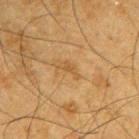Q: Was a biopsy performed?
A: catalogued during a skin exam; not biopsied
Q: Lesion size?
A: ~2.5 mm (longest diameter)
Q: What kind of image is this?
A: 15 mm crop, total-body photography
Q: Who is the patient?
A: male, aged 63–67
Q: Automated lesion metrics?
A: an area of roughly 2.5 mm²; roughly 6 lightness units darker than nearby skin; lesion-presence confidence of about 100/100
Q: Where on the body is the lesion?
A: the right upper arm
Q: What lighting was used for the tile?
A: cross-polarized